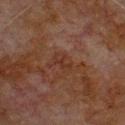{
  "biopsy_status": "not biopsied; imaged during a skin examination",
  "site": "chest",
  "lesion_size": {
    "long_diameter_mm_approx": 2.5
  },
  "patient": {
    "sex": "male",
    "age_approx": 80
  },
  "lighting": "cross-polarized",
  "image": {
    "source": "total-body photography crop",
    "field_of_view_mm": 15
  }
}